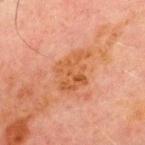The lesion was tiled from a total-body skin photograph and was not biopsied. The lesion is on the chest. Captured under cross-polarized illumination. A male subject, aged 68 to 72. A roughly 15 mm field-of-view crop from a total-body skin photograph. Approximately 5 mm at its widest.